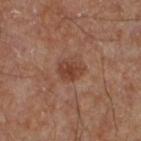This lesion was catalogued during total-body skin photography and was not selected for biopsy.
The lesion is on the left lower leg.
The lesion-visualizer software estimated a mean CIELAB color near L≈41 a*≈22 b*≈28 and roughly 8 lightness units darker than nearby skin. The analysis additionally found a border-irregularity index near 1.5/10, a color-variation rating of about 3/10, and a peripheral color-asymmetry measure near 1. The analysis additionally found an automated nevus-likeness rating near 40 out of 100.
A roughly 15 mm field-of-view crop from a total-body skin photograph.
A male subject, aged 53 to 57.